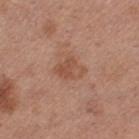notes=no biopsy performed (imaged during a skin exam) | subject=female, about 30 years old | anatomic site=the left thigh | diameter=~3.5 mm (longest diameter) | acquisition=total-body-photography crop, ~15 mm field of view.